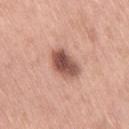The lesion was photographed on a routine skin check and not biopsied; there is no pathology result.
This is a white-light tile.
About 4 mm across.
From the leg.
A close-up tile cropped from a whole-body skin photograph, about 15 mm across.
The subject is a female in their mid-50s.